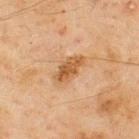Captured during whole-body skin photography for melanoma surveillance; the lesion was not biopsied. The patient is a male aged around 45. Longest diameter approximately 4 mm. Located on the upper back. Captured under cross-polarized illumination. A 15 mm close-up tile from a total-body photography series done for melanoma screening.